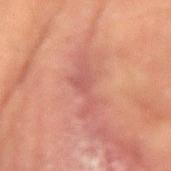biopsy status=catalogued during a skin exam; not biopsied
automated lesion analysis=a mean CIELAB color near L≈47 a*≈24 b*≈23, a lesion–skin lightness drop of about 6, and a normalized border contrast of about 5.5; a lesion-detection confidence of about 75/100
lesion diameter=about 5.5 mm
patient=male, aged approximately 65
imaging modality=total-body-photography crop, ~15 mm field of view
tile lighting=cross-polarized illumination
site=the left upper arm